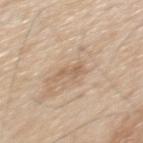Findings:
• imaging modality — ~15 mm crop, total-body skin-cancer survey
• diameter — ≈2.5 mm
• location — the mid back
• patient — male, aged around 60
• illumination — white-light illumination